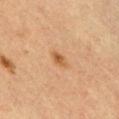Imaged during a routine full-body skin examination; the lesion was not biopsied and no histopathology is available.
Located on the chest.
A male patient approximately 35 years of age.
Imaged with cross-polarized lighting.
A roughly 15 mm field-of-view crop from a total-body skin photograph.